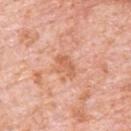workup: catalogued during a skin exam; not biopsied
anatomic site: the upper back
lighting: white-light
imaging modality: ~15 mm crop, total-body skin-cancer survey
automated lesion analysis: a lesion color around L≈62 a*≈26 b*≈35 in CIELAB, a lesion–skin lightness drop of about 8, and a normalized lesion–skin contrast near 5.5; an automated nevus-likeness rating near 0 out of 100 and a lesion-detection confidence of about 100/100
patient: male, about 80 years old